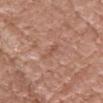Part of a total-body skin-imaging series; this lesion was reviewed on a skin check and was not flagged for biopsy. From the head or neck. An algorithmic analysis of the crop reported an area of roughly 2.5 mm² and a shape eccentricity near 0.9. And it measured a border-irregularity index near 5/10, internal color variation of about 0 on a 0–10 scale, and peripheral color asymmetry of about 0. This is a white-light tile. This image is a 15 mm lesion crop taken from a total-body photograph. About 2.5 mm across. The patient is a female aged 68 to 72.